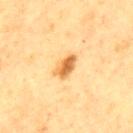The lesion was tiled from a total-body skin photograph and was not biopsied. A male subject aged 73 to 77. This image is a 15 mm lesion crop taken from a total-body photograph. The recorded lesion diameter is about 3.5 mm. This is a cross-polarized tile. The lesion is on the mid back. An algorithmic analysis of the crop reported a lesion area of about 5 mm², an eccentricity of roughly 0.85, and two-axis asymmetry of about 0.25. It also reported roughly 13 lightness units darker than nearby skin and a normalized lesion–skin contrast near 9. And it measured a border-irregularity index near 2.5/10, a within-lesion color-variation index near 3.5/10, and radial color variation of about 1. And it measured an automated nevus-likeness rating near 95 out of 100 and a detector confidence of about 100 out of 100 that the crop contains a lesion.